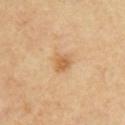{"biopsy_status": "not biopsied; imaged during a skin examination", "image": {"source": "total-body photography crop", "field_of_view_mm": 15}, "automated_metrics": {"area_mm2_approx": 4.0, "eccentricity": 0.55, "shape_asymmetry": 0.3, "nevus_likeness_0_100": 60, "lesion_detection_confidence_0_100": 100}, "site": "right upper arm", "lesion_size": {"long_diameter_mm_approx": 2.0}, "patient": {"sex": "male", "age_approx": 55}, "lighting": "cross-polarized"}